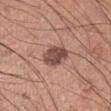Notes:
• size: ≈3.5 mm
• site: the right upper arm
• acquisition: total-body-photography crop, ~15 mm field of view
• illumination: white-light
• automated metrics: a lesion area of about 7.5 mm², a shape eccentricity near 0.7, and a shape-asymmetry score of about 0.2 (0 = symmetric); a lesion–skin lightness drop of about 14 and a lesion-to-skin contrast of about 10.5 (normalized; higher = more distinct); a border-irregularity index near 2/10, a within-lesion color-variation index near 3.5/10, and a peripheral color-asymmetry measure near 1
• subject: male, aged around 35